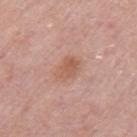Clinical impression:
Captured during whole-body skin photography for melanoma surveillance; the lesion was not biopsied.